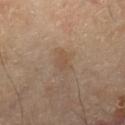Q: Was this lesion biopsied?
A: total-body-photography surveillance lesion; no biopsy
Q: What is the anatomic site?
A: the right lower leg
Q: How was this image acquired?
A: ~15 mm tile from a whole-body skin photo
Q: Patient demographics?
A: female, aged around 70
Q: What did automated image analysis measure?
A: a lesion area of about 3 mm², a shape eccentricity near 0.75, and a shape-asymmetry score of about 0.25 (0 = symmetric); a within-lesion color-variation index near 1.5/10 and radial color variation of about 0.5; an automated nevus-likeness rating near 0 out of 100 and a detector confidence of about 100 out of 100 that the crop contains a lesion
Q: Illumination type?
A: cross-polarized
Q: How large is the lesion?
A: ≈2.5 mm